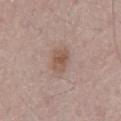<tbp_lesion>
  <biopsy_status>not biopsied; imaged during a skin examination</biopsy_status>
  <patient>
    <sex>male</sex>
    <age_approx>65</age_approx>
  </patient>
  <lighting>white-light</lighting>
  <automated_metrics>
    <area_mm2_approx>6.0</area_mm2_approx>
    <shape_asymmetry>0.2</shape_asymmetry>
  </automated_metrics>
  <site>abdomen</site>
  <image>
    <source>total-body photography crop</source>
    <field_of_view_mm>15</field_of_view_mm>
  </image>
</tbp_lesion>